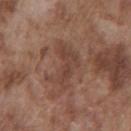Image and clinical context: A region of skin cropped from a whole-body photographic capture, roughly 15 mm wide. The lesion is located on the chest. The subject is a male approximately 75 years of age. The lesion's longest dimension is about 5.5 mm.